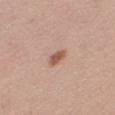notes: total-body-photography surveillance lesion; no biopsy | lesion diameter: about 2.5 mm | illumination: white-light | subject: female, approximately 40 years of age | anatomic site: the right thigh | image source: ~15 mm crop, total-body skin-cancer survey.